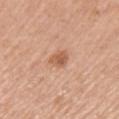follow-up: catalogued during a skin exam; not biopsied | size: ≈3 mm | lighting: white-light | patient: male, in their 70s | anatomic site: the back | imaging modality: ~15 mm tile from a whole-body skin photo.